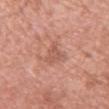The lesion was tiled from a total-body skin photograph and was not biopsied. Captured under white-light illumination. A 15 mm close-up tile from a total-body photography series done for melanoma screening. From the upper back. A female patient, about 60 years old. Longest diameter approximately 3.5 mm. The total-body-photography lesion software estimated an outline eccentricity of about 0.65 (0 = round, 1 = elongated) and two-axis asymmetry of about 0.5. The analysis additionally found a border-irregularity index near 5/10, internal color variation of about 2.5 on a 0–10 scale, and a peripheral color-asymmetry measure near 1.